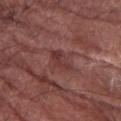<record>
<biopsy_status>not biopsied; imaged during a skin examination</biopsy_status>
<site>right forearm</site>
<image>
  <source>total-body photography crop</source>
  <field_of_view_mm>15</field_of_view_mm>
</image>
<patient>
  <sex>male</sex>
  <age_approx>75</age_approx>
</patient>
<lighting>white-light</lighting>
<lesion_size>
  <long_diameter_mm_approx>4.0</long_diameter_mm_approx>
</lesion_size>
</record>